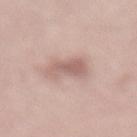biopsy status: no biopsy performed (imaged during a skin exam)
illumination: white-light
TBP lesion metrics: an eccentricity of roughly 0.65 and a symmetry-axis asymmetry near 0.35; a lesion color around L≈60 a*≈19 b*≈22 in CIELAB, a lesion–skin lightness drop of about 10, and a lesion-to-skin contrast of about 6.5 (normalized; higher = more distinct); a border-irregularity rating of about 4/10, internal color variation of about 2.5 on a 0–10 scale, and radial color variation of about 0.5
acquisition: total-body-photography crop, ~15 mm field of view
body site: the lower back
diameter: about 3.5 mm
patient: male, in their 50s